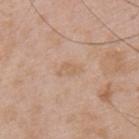biopsy status=total-body-photography surveillance lesion; no biopsy | automated lesion analysis=an average lesion color of about L≈62 a*≈17 b*≈31 (CIELAB), roughly 6 lightness units darker than nearby skin, and a normalized border contrast of about 4.5 | patient=male, aged around 50 | tile lighting=white-light | anatomic site=the upper back | size=≈3 mm | imaging modality=total-body-photography crop, ~15 mm field of view.